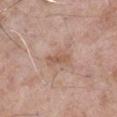acquisition: ~15 mm tile from a whole-body skin photo | patient: male, in their mid-70s | size: ~3 mm (longest diameter) | location: the leg | automated lesion analysis: a footprint of about 4.5 mm², an outline eccentricity of about 0.85 (0 = round, 1 = elongated), and two-axis asymmetry of about 0.3; a peripheral color-asymmetry measure near 0.5 | illumination: white-light illumination.